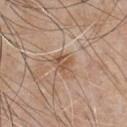follow-up=total-body-photography surveillance lesion; no biopsy
site=the chest
imaging modality=~15 mm crop, total-body skin-cancer survey
subject=male, approximately 65 years of age
illumination=white-light illumination
diameter=≈3 mm